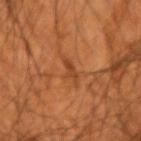notes: total-body-photography surveillance lesion; no biopsy
location: the left forearm
TBP lesion metrics: a footprint of about 2.5 mm², an eccentricity of roughly 0.85, and two-axis asymmetry of about 0.35; a nevus-likeness score of about 0/100
lighting: cross-polarized illumination
image: 15 mm crop, total-body photography
subject: male, aged approximately 55
diameter: ≈2.5 mm